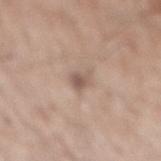workup = no biopsy performed (imaged during a skin exam); subject = male, in their 60s; lighting = white-light; diameter = ≈2.5 mm; image = ~15 mm tile from a whole-body skin photo; body site = the mid back.